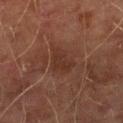workup: catalogued during a skin exam; not biopsied | subject: male, aged 73 to 77 | lesion diameter: ≈4.5 mm | location: the right lower leg | imaging modality: ~15 mm crop, total-body skin-cancer survey | automated lesion analysis: an average lesion color of about L≈26 a*≈17 b*≈22 (CIELAB) and a lesion–skin lightness drop of about 5; a classifier nevus-likeness of about 0/100 and a detector confidence of about 100 out of 100 that the crop contains a lesion | lighting: cross-polarized illumination.